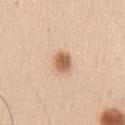workup: imaged on a skin check; not biopsied
anatomic site: the left upper arm
image-analysis metrics: a lesion color around L≈63 a*≈20 b*≈35 in CIELAB and roughly 14 lightness units darker than nearby skin; border irregularity of about 1.5 on a 0–10 scale, a color-variation rating of about 4/10, and radial color variation of about 1.5; a nevus-likeness score of about 100/100
image source: ~15 mm crop, total-body skin-cancer survey
patient: male, aged approximately 60
tile lighting: white-light illumination